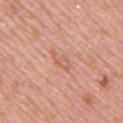Clinical impression:
Imaged during a routine full-body skin examination; the lesion was not biopsied and no histopathology is available.
Image and clinical context:
Imaged with white-light lighting. A lesion tile, about 15 mm wide, cut from a 3D total-body photograph. The lesion is on the upper back. The lesion's longest dimension is about 3.5 mm. An algorithmic analysis of the crop reported a mean CIELAB color near L≈61 a*≈24 b*≈32, roughly 7 lightness units darker than nearby skin, and a normalized lesion–skin contrast near 5. And it measured an automated nevus-likeness rating near 0 out of 100 and a detector confidence of about 100 out of 100 that the crop contains a lesion. A male patient aged approximately 55.